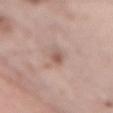Q: Is there a histopathology result?
A: total-body-photography surveillance lesion; no biopsy
Q: Automated lesion metrics?
A: a within-lesion color-variation index near 2/10 and radial color variation of about 0.5; an automated nevus-likeness rating near 0 out of 100 and a lesion-detection confidence of about 100/100
Q: How was this image acquired?
A: 15 mm crop, total-body photography
Q: How large is the lesion?
A: about 3.5 mm
Q: Illumination type?
A: white-light illumination
Q: Where on the body is the lesion?
A: the mid back
Q: Patient demographics?
A: male, in their mid- to late 60s